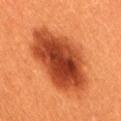biopsy status = no biopsy performed (imaged during a skin exam) | subject = female, approximately 30 years of age | image source = 15 mm crop, total-body photography | site = the lower back.